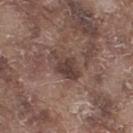The lesion was tiled from a total-body skin photograph and was not biopsied. A 15 mm close-up tile from a total-body photography series done for melanoma screening. Longest diameter approximately 4.5 mm. The lesion is located on the right thigh. The tile uses white-light illumination. The subject is a male aged approximately 75.